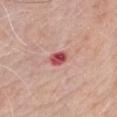– notes — catalogued during a skin exam; not biopsied
– patient — male, aged around 80
– body site — the chest
– acquisition — ~15 mm crop, total-body skin-cancer survey